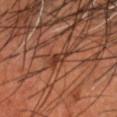  biopsy_status: not biopsied; imaged during a skin examination
  image:
    source: total-body photography crop
    field_of_view_mm: 15
  lighting: cross-polarized
  patient:
    sex: male
    age_approx: 55
  site: head or neck
  automated_metrics:
    cielab_L: 34
    cielab_a: 24
    cielab_b: 29
    vs_skin_contrast_norm: 8.5
    color_variation_0_10: 0.0
    peripheral_color_asymmetry: 0.0
    nevus_likeness_0_100: 0
    lesion_detection_confidence_0_100: 35